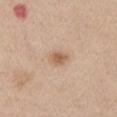• notes — catalogued during a skin exam; not biopsied
• image — 15 mm crop, total-body photography
• lesion diameter — ≈2.5 mm
• illumination — white-light
• subject — male, approximately 60 years of age
• site — the left upper arm